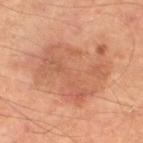The lesion was photographed on a routine skin check and not biopsied; there is no pathology result.
The lesion is located on the left lower leg.
A male subject in their 50s.
This image is a 15 mm lesion crop taken from a total-body photograph.
Longest diameter approximately 9 mm.